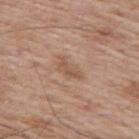Imaged during a routine full-body skin examination; the lesion was not biopsied and no histopathology is available. Located on the back. The tile uses white-light illumination. This image is a 15 mm lesion crop taken from a total-body photograph. About 3.5 mm across. A male subject aged 68 to 72.